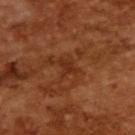Clinical impression:
Part of a total-body skin-imaging series; this lesion was reviewed on a skin check and was not flagged for biopsy.
Background:
Captured under cross-polarized illumination. The recorded lesion diameter is about 2.5 mm. A male subject, aged approximately 65. Cropped from a total-body skin-imaging series; the visible field is about 15 mm.